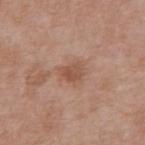The lesion was tiled from a total-body skin photograph and was not biopsied. Measured at roughly 2.5 mm in maximum diameter. An algorithmic analysis of the crop reported a lesion color around L≈52 a*≈21 b*≈29 in CIELAB. It also reported border irregularity of about 2.5 on a 0–10 scale, internal color variation of about 3.5 on a 0–10 scale, and radial color variation of about 1. The software also gave a classifier nevus-likeness of about 20/100 and lesion-presence confidence of about 100/100. The lesion is located on the abdomen. The tile uses white-light illumination. Cropped from a whole-body photographic skin survey; the tile spans about 15 mm. A male patient roughly 70 years of age.